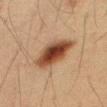<record>
  <biopsy_status>not biopsied; imaged during a skin examination</biopsy_status>
  <site>right thigh</site>
  <image>
    <source>total-body photography crop</source>
    <field_of_view_mm>15</field_of_view_mm>
  </image>
  <patient>
    <sex>male</sex>
    <age_approx>40</age_approx>
  </patient>
</record>